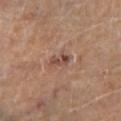The lesion was tiled from a total-body skin photograph and was not biopsied.
Approximately 2.5 mm at its widest.
On the leg.
The tile uses cross-polarized illumination.
A roughly 15 mm field-of-view crop from a total-body skin photograph.
A female patient, roughly 65 years of age.